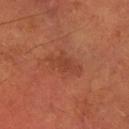tile lighting=cross-polarized illumination; body site=the right forearm; image=15 mm crop, total-body photography; diameter=about 4 mm; patient=male, approximately 65 years of age.